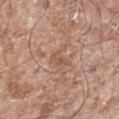This lesion was catalogued during total-body skin photography and was not selected for biopsy.
A male patient aged around 60.
Longest diameter approximately 3 mm.
A 15 mm close-up tile from a total-body photography series done for melanoma screening.
Located on the right lower leg.